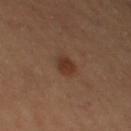| feature | finding |
|---|---|
| workup | imaged on a skin check; not biopsied |
| tile lighting | cross-polarized illumination |
| patient | female, aged around 60 |
| body site | the left thigh |
| acquisition | ~15 mm crop, total-body skin-cancer survey |
| TBP lesion metrics | a shape eccentricity near 0.35 and a shape-asymmetry score of about 0.2 (0 = symmetric); lesion-presence confidence of about 100/100 |
| lesion size | ~2.5 mm (longest diameter) |